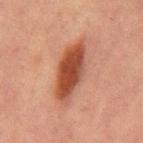patient = male, aged around 65
acquisition = ~15 mm crop, total-body skin-cancer survey
location = the abdomen
tile lighting = cross-polarized